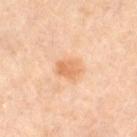body site = the right thigh
diameter = ~2.5 mm (longest diameter)
image = ~15 mm tile from a whole-body skin photo
patient = female, about 50 years old
lighting = cross-polarized